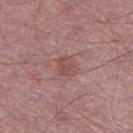Clinical impression: Imaged during a routine full-body skin examination; the lesion was not biopsied and no histopathology is available. Context: A close-up tile cropped from a whole-body skin photograph, about 15 mm across. Longest diameter approximately 2.5 mm. A male patient, aged 63 to 67. On the leg.